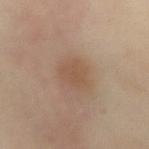site = the front of the torso
subject = female, roughly 60 years of age
tile lighting = cross-polarized
size = ≈3 mm
image = ~15 mm tile from a whole-body skin photo